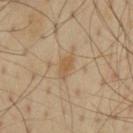Q: Was a biopsy performed?
A: no biopsy performed (imaged during a skin exam)
Q: What is the imaging modality?
A: 15 mm crop, total-body photography
Q: Lesion location?
A: the mid back
Q: Patient demographics?
A: male, aged 53 to 57
Q: What is the lesion's diameter?
A: about 3.5 mm